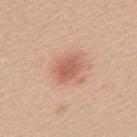workup: no biopsy performed (imaged during a skin exam); body site: the left upper arm; image source: ~15 mm tile from a whole-body skin photo; lighting: white-light; subject: female, about 25 years old; size: ~3 mm (longest diameter).